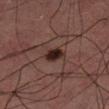<record>
<biopsy_status>not biopsied; imaged during a skin examination</biopsy_status>
<lighting>cross-polarized</lighting>
<lesion_size>
  <long_diameter_mm_approx>2.5</long_diameter_mm_approx>
</lesion_size>
<image>
  <source>total-body photography crop</source>
  <field_of_view_mm>15</field_of_view_mm>
</image>
<patient>
  <sex>male</sex>
  <age_approx>60</age_approx>
</patient>
</record>